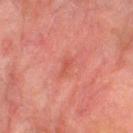Findings:
– workup · imaged on a skin check; not biopsied
– lesion size · ≈2.5 mm
– image source · 15 mm crop, total-body photography
– automated lesion analysis · a lesion area of about 2.5 mm², an outline eccentricity of about 0.9 (0 = round, 1 = elongated), and a shape-asymmetry score of about 0.25 (0 = symmetric)
– patient · male, about 75 years old
– tile lighting · cross-polarized illumination
– anatomic site · the leg